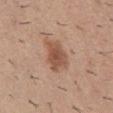Recorded during total-body skin imaging; not selected for excision or biopsy. This is a white-light tile. The subject is a male aged around 30. A 15 mm crop from a total-body photograph taken for skin-cancer surveillance. The lesion-visualizer software estimated a lesion color around L≈52 a*≈21 b*≈30 in CIELAB and a normalized border contrast of about 8. The software also gave a border-irregularity rating of about 3/10, internal color variation of about 3 on a 0–10 scale, and a peripheral color-asymmetry measure near 1. From the abdomen.